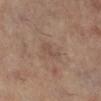biopsy status: catalogued during a skin exam; not biopsied | illumination: cross-polarized | acquisition: ~15 mm tile from a whole-body skin photo | TBP lesion metrics: a classifier nevus-likeness of about 0/100 and a lesion-detection confidence of about 100/100 | anatomic site: the right lower leg | patient: female, aged 58–62 | lesion diameter: about 3.5 mm.